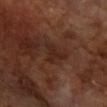Q: Is there a histopathology result?
A: no biopsy performed (imaged during a skin exam)
Q: How was this image acquired?
A: total-body-photography crop, ~15 mm field of view
Q: Lesion location?
A: the right forearm
Q: What are the patient's age and sex?
A: female, aged around 65